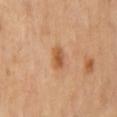Recorded during total-body skin imaging; not selected for excision or biopsy.
A lesion tile, about 15 mm wide, cut from a 3D total-body photograph.
The lesion-visualizer software estimated a lesion area of about 4.5 mm² and an outline eccentricity of about 0.8 (0 = round, 1 = elongated). And it measured a lesion color around L≈57 a*≈24 b*≈39 in CIELAB, roughly 10 lightness units darker than nearby skin, and a normalized border contrast of about 7.5. The analysis additionally found a border-irregularity index near 2/10, a color-variation rating of about 3/10, and peripheral color asymmetry of about 1. The software also gave an automated nevus-likeness rating near 80 out of 100 and a lesion-detection confidence of about 100/100.
Located on the mid back.
Measured at roughly 3 mm in maximum diameter.
This is a cross-polarized tile.